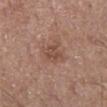Q: Is there a histopathology result?
A: no biopsy performed (imaged during a skin exam)
Q: What lighting was used for the tile?
A: white-light
Q: What is the imaging modality?
A: total-body-photography crop, ~15 mm field of view
Q: What is the lesion's diameter?
A: ~3.5 mm (longest diameter)
Q: Where on the body is the lesion?
A: the right lower leg
Q: Who is the patient?
A: male, approximately 60 years of age
Q: Automated lesion metrics?
A: an average lesion color of about L≈49 a*≈20 b*≈26 (CIELAB) and a normalized lesion–skin contrast near 6; a classifier nevus-likeness of about 5/100 and a detector confidence of about 100 out of 100 that the crop contains a lesion